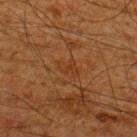Notes:
• notes — catalogued during a skin exam; not biopsied
• anatomic site — the upper back
• patient — male, aged 63–67
• image source — ~15 mm tile from a whole-body skin photo
• automated metrics — an eccentricity of roughly 0.8 and two-axis asymmetry of about 0.45; a mean CIELAB color near L≈30 a*≈19 b*≈31, roughly 4 lightness units darker than nearby skin, and a normalized border contrast of about 4.5; an automated nevus-likeness rating near 0 out of 100
• diameter — ~2.5 mm (longest diameter)